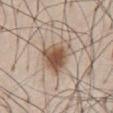Recorded during total-body skin imaging; not selected for excision or biopsy.
Cropped from a total-body skin-imaging series; the visible field is about 15 mm.
The lesion is on the front of the torso.
A male patient aged approximately 60.